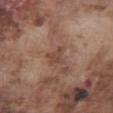Part of a total-body skin-imaging series; this lesion was reviewed on a skin check and was not flagged for biopsy.
Cropped from a total-body skin-imaging series; the visible field is about 15 mm.
From the abdomen.
Captured under white-light illumination.
The recorded lesion diameter is about 2.5 mm.
The subject is a male aged around 75.